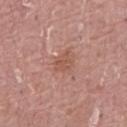Case summary:
- biopsy status — catalogued during a skin exam; not biopsied
- patient — male, aged around 40
- lighting — white-light
- diameter — ≈2.5 mm
- body site — the abdomen
- imaging modality — ~15 mm tile from a whole-body skin photo